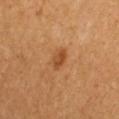{"patient": {"sex": "female", "age_approx": 30}, "image": {"source": "total-body photography crop", "field_of_view_mm": 15}, "site": "chest", "automated_metrics": {"area_mm2_approx": 3.0, "eccentricity": 0.85, "shape_asymmetry": 0.25, "nevus_likeness_0_100": 65}, "lighting": "cross-polarized", "lesion_size": {"long_diameter_mm_approx": 2.5}}